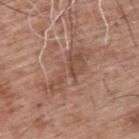Cropped from a whole-body photographic skin survey; the tile spans about 15 mm.
Approximately 7 mm at its widest.
On the upper back.
The tile uses white-light illumination.
A male patient roughly 60 years of age.